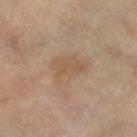The lesion was photographed on a routine skin check and not biopsied; there is no pathology result. About 4.5 mm across. The lesion is on the left lower leg. A 15 mm crop from a total-body photograph taken for skin-cancer surveillance. The subject is aged approximately 60.